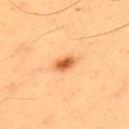Q: Automated lesion metrics?
A: a footprint of about 4 mm² and an outline eccentricity of about 0.7 (0 = round, 1 = elongated); border irregularity of about 1 on a 0–10 scale and a peripheral color-asymmetry measure near 1.5
Q: What is the lesion's diameter?
A: ~2.5 mm (longest diameter)
Q: How was this image acquired?
A: ~15 mm tile from a whole-body skin photo
Q: What is the anatomic site?
A: the upper back
Q: How was the tile lit?
A: cross-polarized illumination
Q: What are the patient's age and sex?
A: male, aged 53 to 57